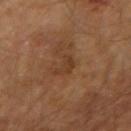follow-up: total-body-photography surveillance lesion; no biopsy
subject: male, roughly 70 years of age
body site: the right upper arm
diameter: ~2.5 mm (longest diameter)
illumination: cross-polarized illumination
image source: total-body-photography crop, ~15 mm field of view